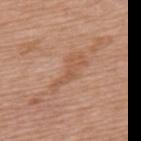The lesion was tiled from a total-body skin photograph and was not biopsied. A close-up tile cropped from a whole-body skin photograph, about 15 mm across. On the upper back. The lesion-visualizer software estimated a footprint of about 7 mm² and an eccentricity of roughly 0.95. The analysis additionally found a lesion color around L≈55 a*≈21 b*≈33 in CIELAB, roughly 7 lightness units darker than nearby skin, and a normalized lesion–skin contrast near 5.5. The analysis additionally found a nevus-likeness score of about 0/100 and a detector confidence of about 55 out of 100 that the crop contains a lesion. Longest diameter approximately 5.5 mm. The tile uses white-light illumination. The subject is a male about 80 years old.